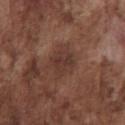Imaged during a routine full-body skin examination; the lesion was not biopsied and no histopathology is available.
Cropped from a total-body skin-imaging series; the visible field is about 15 mm.
The lesion is on the chest.
A male subject, about 75 years old.
The lesion's longest dimension is about 3.5 mm.
This is a white-light tile.